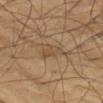biopsy status: no biopsy performed (imaged during a skin exam) | size: about 4 mm | illumination: cross-polarized illumination | patient: male, approximately 65 years of age | image: 15 mm crop, total-body photography | anatomic site: the chest | automated metrics: about 6 CIELAB-L* units darker than the surrounding skin and a normalized border contrast of about 5; a nevus-likeness score of about 0/100 and a detector confidence of about 60 out of 100 that the crop contains a lesion.